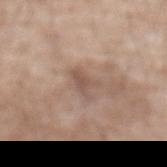Impression: The lesion was tiled from a total-body skin photograph and was not biopsied. Acquisition and patient details: Longest diameter approximately 3.5 mm. The lesion is located on the abdomen. A close-up tile cropped from a whole-body skin photograph, about 15 mm across. Captured under white-light illumination. A male subject, aged 68 to 72.